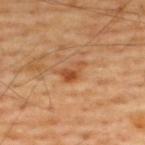Part of a total-body skin-imaging series; this lesion was reviewed on a skin check and was not flagged for biopsy.